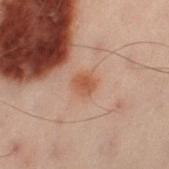Recorded during total-body skin imaging; not selected for excision or biopsy.
A 15 mm close-up tile from a total-body photography series done for melanoma screening.
On the left thigh.
The patient is a male about 50 years old.